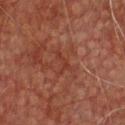acquisition = ~15 mm crop, total-body skin-cancer survey | subject = male, approximately 75 years of age | lighting = cross-polarized illumination | location = the chest | automated lesion analysis = a lesion area of about 2.5 mm², a shape eccentricity near 0.85, and two-axis asymmetry of about 0.7; an average lesion color of about L≈30 a*≈23 b*≈25 (CIELAB) and a lesion–skin lightness drop of about 5; a color-variation rating of about 0/10 and peripheral color asymmetry of about 0; a nevus-likeness score of about 0/100 | lesion size = ≈3 mm.